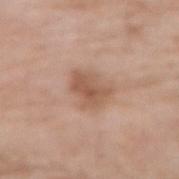{"site": "left forearm", "patient": {"sex": "female", "age_approx": "85+"}, "lighting": "white-light", "lesion_size": {"long_diameter_mm_approx": 3.5}, "image": {"source": "total-body photography crop", "field_of_view_mm": 15}}